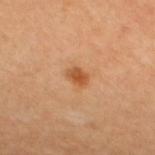Recorded during total-body skin imaging; not selected for excision or biopsy.
Located on the upper back.
The tile uses cross-polarized illumination.
Automated tile analysis of the lesion measured a footprint of about 3.5 mm², a shape eccentricity near 0.65, and two-axis asymmetry of about 0.2. The analysis additionally found a lesion color around L≈55 a*≈26 b*≈41 in CIELAB, a lesion–skin lightness drop of about 11, and a normalized border contrast of about 8. And it measured internal color variation of about 2.5 on a 0–10 scale and radial color variation of about 1.
A 15 mm crop from a total-body photograph taken for skin-cancer surveillance.
The lesion's longest dimension is about 2.5 mm.
A female patient approximately 30 years of age.